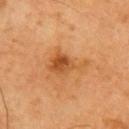Impression: The lesion was photographed on a routine skin check and not biopsied; there is no pathology result. Context: A male patient, roughly 70 years of age. Automated tile analysis of the lesion measured a footprint of about 10 mm² and an outline eccentricity of about 0.85 (0 = round, 1 = elongated). It also reported a lesion color around L≈51 a*≈25 b*≈41 in CIELAB and a lesion–skin lightness drop of about 10. It also reported a border-irregularity rating of about 6/10, a color-variation rating of about 6.5/10, and peripheral color asymmetry of about 2. The analysis additionally found an automated nevus-likeness rating near 60 out of 100. This is a cross-polarized tile. Longest diameter approximately 5 mm. From the right upper arm. A 15 mm close-up extracted from a 3D total-body photography capture.